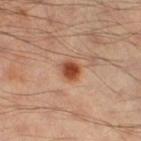Q: Was a biopsy performed?
A: total-body-photography surveillance lesion; no biopsy
Q: Patient demographics?
A: male, in their mid-50s
Q: What did automated image analysis measure?
A: roughly 14 lightness units darker than nearby skin
Q: Where on the body is the lesion?
A: the leg
Q: Lesion size?
A: about 2.5 mm
Q: What lighting was used for the tile?
A: cross-polarized illumination
Q: What is the imaging modality?
A: 15 mm crop, total-body photography